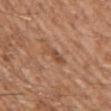Q: Was this lesion biopsied?
A: imaged on a skin check; not biopsied
Q: What are the patient's age and sex?
A: male, aged 58 to 62
Q: What did automated image analysis measure?
A: a footprint of about 3.5 mm², a shape eccentricity near 0.9, and a shape-asymmetry score of about 0.3 (0 = symmetric)
Q: What is the anatomic site?
A: the left upper arm
Q: How was this image acquired?
A: ~15 mm crop, total-body skin-cancer survey
Q: Illumination type?
A: white-light